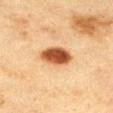| key | value |
|---|---|
| follow-up | total-body-photography surveillance lesion; no biopsy |
| illumination | cross-polarized |
| anatomic site | the leg |
| patient | female, about 40 years old |
| size | ~4 mm (longest diameter) |
| automated metrics | a footprint of about 9.5 mm², an eccentricity of roughly 0.6, and a shape-asymmetry score of about 0.1 (0 = symmetric); border irregularity of about 1 on a 0–10 scale and a within-lesion color-variation index near 5.5/10; a classifier nevus-likeness of about 100/100 |
| imaging modality | total-body-photography crop, ~15 mm field of view |